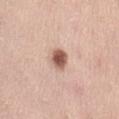Impression: Captured during whole-body skin photography for melanoma surveillance; the lesion was not biopsied. Clinical summary: A female subject, aged approximately 40. The tile uses white-light illumination. A region of skin cropped from a whole-body photographic capture, roughly 15 mm wide. Located on the left thigh. The lesion's longest dimension is about 2.5 mm. The lesion-visualizer software estimated an average lesion color of about L≈56 a*≈21 b*≈27 (CIELAB) and a normalized lesion–skin contrast near 11. The analysis additionally found a detector confidence of about 100 out of 100 that the crop contains a lesion.